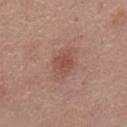Findings:
- biopsy status: catalogued during a skin exam; not biopsied
- image source: total-body-photography crop, ~15 mm field of view
- lesion size: ≈3 mm
- subject: male, aged around 55
- site: the mid back